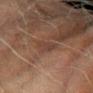Impression:
The lesion was photographed on a routine skin check and not biopsied; there is no pathology result.
Image and clinical context:
A male patient, aged around 70. An algorithmic analysis of the crop reported an outline eccentricity of about 0.6 (0 = round, 1 = elongated) and two-axis asymmetry of about 0.2. And it measured a lesion color around L≈32 a*≈15 b*≈22 in CIELAB and roughly 4 lightness units darker than nearby skin. The software also gave a color-variation rating of about 2/10 and peripheral color asymmetry of about 1. A lesion tile, about 15 mm wide, cut from a 3D total-body photograph. On the left forearm. Imaged with cross-polarized lighting.